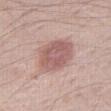Impression:
Imaged during a routine full-body skin examination; the lesion was not biopsied and no histopathology is available.
Image and clinical context:
A close-up tile cropped from a whole-body skin photograph, about 15 mm across. The patient is a male aged 63 to 67. Located on the right thigh. Measured at roughly 5 mm in maximum diameter. Captured under white-light illumination.